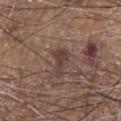No biopsy was performed on this lesion — it was imaged during a full skin examination and was not determined to be concerning. Cropped from a total-body skin-imaging series; the visible field is about 15 mm. The tile uses white-light illumination. Located on the chest. Longest diameter approximately 3.5 mm. A male subject aged 78–82. The lesion-visualizer software estimated a lesion area of about 4.5 mm². The analysis additionally found a lesion color around L≈39 a*≈15 b*≈21 in CIELAB, roughly 8 lightness units darker than nearby skin, and a lesion-to-skin contrast of about 7 (normalized; higher = more distinct). It also reported a classifier nevus-likeness of about 0/100.